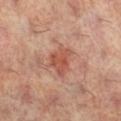illumination: cross-polarized illumination | subject: female, aged 58 to 62 | automated metrics: a lesion area of about 7 mm², a shape eccentricity near 0.6, and a shape-asymmetry score of about 0.35 (0 = symmetric); about 10 CIELAB-L* units darker than the surrounding skin and a normalized border contrast of about 7; a border-irregularity index near 3.5/10, internal color variation of about 4 on a 0–10 scale, and radial color variation of about 1.5 | location: the right lower leg | acquisition: 15 mm crop, total-body photography.